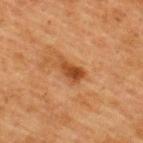Recorded during total-body skin imaging; not selected for excision or biopsy. This is a cross-polarized tile. The lesion's longest dimension is about 3.5 mm. The lesion is on the upper back. A region of skin cropped from a whole-body photographic capture, roughly 15 mm wide. A female subject aged 38–42.